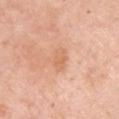The total-body-photography lesion software estimated a footprint of about 4.5 mm², an eccentricity of roughly 0.7, and two-axis asymmetry of about 0.25. The software also gave a color-variation rating of about 2/10 and radial color variation of about 0.5. The analysis additionally found a nevus-likeness score of about 0/100 and lesion-presence confidence of about 100/100.
The tile uses white-light illumination.
Longest diameter approximately 3 mm.
A female patient, approximately 65 years of age.
Cropped from a total-body skin-imaging series; the visible field is about 15 mm.
On the arm.